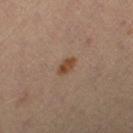  biopsy_status: not biopsied; imaged during a skin examination
  site: leg
  patient:
    sex: female
    age_approx: 45
  image:
    source: total-body photography crop
    field_of_view_mm: 15